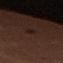The lesion was photographed on a routine skin check and not biopsied; there is no pathology result.
A female subject, aged approximately 70.
The tile uses cross-polarized illumination.
The lesion's longest dimension is about 3 mm.
A 15 mm close-up tile from a total-body photography series done for melanoma screening.
Automated image analysis of the tile measured an area of roughly 3 mm², an eccentricity of roughly 0.9, and a shape-asymmetry score of about 0.3 (0 = symmetric). And it measured a lesion color around L≈15 a*≈14 b*≈15 in CIELAB, roughly 4 lightness units darker than nearby skin, and a normalized lesion–skin contrast near 6. The software also gave a border-irregularity rating of about 3/10, a color-variation rating of about 2.5/10, and radial color variation of about 1. It also reported a classifier nevus-likeness of about 0/100.
The lesion is on the left thigh.